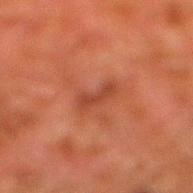subject: male, about 80 years old
image-analysis metrics: a footprint of about 3.5 mm² and a shape-asymmetry score of about 0.5 (0 = symmetric); a border-irregularity index near 6/10, internal color variation of about 0 on a 0–10 scale, and a peripheral color-asymmetry measure near 0; a nevus-likeness score of about 0/100
size: about 3.5 mm
image: ~15 mm tile from a whole-body skin photo
location: the leg